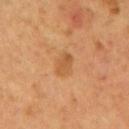<lesion>
<site>mid back</site>
<lighting>cross-polarized</lighting>
<automated_metrics>
  <vs_skin_darker_L>7.0</vs_skin_darker_L>
  <vs_skin_contrast_norm>6.0</vs_skin_contrast_norm>
</automated_metrics>
<patient>
  <sex>male</sex>
  <age_approx>55</age_approx>
</patient>
<lesion_size>
  <long_diameter_mm_approx>3.0</long_diameter_mm_approx>
</lesion_size>
<image>
  <source>total-body photography crop</source>
  <field_of_view_mm>15</field_of_view_mm>
</image>
</lesion>